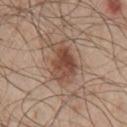| field | value |
|---|---|
| follow-up | catalogued during a skin exam; not biopsied |
| size | about 5.5 mm |
| lighting | white-light illumination |
| subject | male, aged approximately 55 |
| image | total-body-photography crop, ~15 mm field of view |
| anatomic site | the chest |